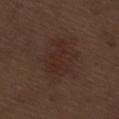{
  "biopsy_status": "not biopsied; imaged during a skin examination",
  "patient": {
    "sex": "male",
    "age_approx": 70
  },
  "lighting": "white-light",
  "image": {
    "source": "total-body photography crop",
    "field_of_view_mm": 15
  },
  "site": "right thigh"
}